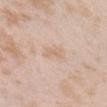Impression: This lesion was catalogued during total-body skin photography and was not selected for biopsy. Context: The lesion is on the head or neck. The lesion-visualizer software estimated a lesion color around L≈68 a*≈16 b*≈30 in CIELAB, a lesion–skin lightness drop of about 6, and a normalized border contrast of about 5. The analysis additionally found a border-irregularity index near 3/10, a color-variation rating of about 1/10, and radial color variation of about 0.5. The lesion's longest dimension is about 2.5 mm. A female subject aged around 25. A close-up tile cropped from a whole-body skin photograph, about 15 mm across.